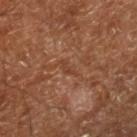Captured during whole-body skin photography for melanoma surveillance; the lesion was not biopsied.